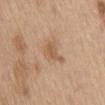Impression:
The lesion was photographed on a routine skin check and not biopsied; there is no pathology result.
Background:
On the mid back. Imaged with white-light lighting. A region of skin cropped from a whole-body photographic capture, roughly 15 mm wide. Approximately 3 mm at its widest. A male subject, aged 68–72. Automated tile analysis of the lesion measured a lesion area of about 5 mm², an eccentricity of roughly 0.7, and a shape-asymmetry score of about 0.5 (0 = symmetric). The software also gave border irregularity of about 5 on a 0–10 scale, a color-variation rating of about 1.5/10, and radial color variation of about 0.5.